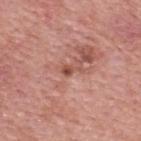{
  "biopsy_status": "not biopsied; imaged during a skin examination",
  "patient": {
    "sex": "female",
    "age_approx": 40
  },
  "site": "upper back",
  "lighting": "white-light",
  "image": {
    "source": "total-body photography crop",
    "field_of_view_mm": 15
  }
}